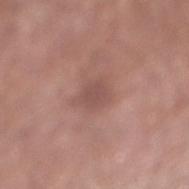{
  "biopsy_status": "not biopsied; imaged during a skin examination",
  "site": "right lower leg",
  "patient": {
    "sex": "male",
    "age_approx": 65
  },
  "image": {
    "source": "total-body photography crop",
    "field_of_view_mm": 15
  },
  "lighting": "white-light",
  "automated_metrics": {
    "nevus_likeness_0_100": 0,
    "lesion_detection_confidence_0_100": 100
  },
  "lesion_size": {
    "long_diameter_mm_approx": 2.5
  }
}